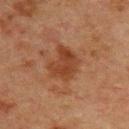notes: imaged on a skin check; not biopsied | location: the upper back | subject: female, aged around 55 | imaging modality: ~15 mm crop, total-body skin-cancer survey.